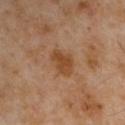follow-up: no biopsy performed (imaged during a skin exam).